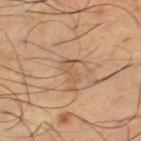- follow-up · no biopsy performed (imaged during a skin exam)
- patient · male, aged approximately 65
- site · the right thigh
- lesion size · ~3 mm (longest diameter)
- imaging modality · total-body-photography crop, ~15 mm field of view
- automated metrics · a mean CIELAB color near L≈56 a*≈18 b*≈33 and about 7 CIELAB-L* units darker than the surrounding skin